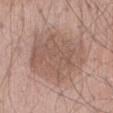location: the abdomen | illumination: white-light | subject: male, aged approximately 65 | diameter: ~7.5 mm (longest diameter) | acquisition: total-body-photography crop, ~15 mm field of view | TBP lesion metrics: an average lesion color of about L≈55 a*≈18 b*≈25 (CIELAB), a lesion–skin lightness drop of about 8, and a lesion-to-skin contrast of about 6 (normalized; higher = more distinct); a border-irregularity index near 3/10, a within-lesion color-variation index near 3.5/10, and radial color variation of about 1; an automated nevus-likeness rating near 0 out of 100 and lesion-presence confidence of about 100/100.